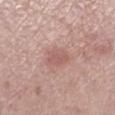– follow-up · total-body-photography surveillance lesion; no biopsy
– diameter · ≈3 mm
– acquisition · total-body-photography crop, ~15 mm field of view
– illumination · white-light
– subject · female, aged approximately 65
– location · the left lower leg
– image-analysis metrics · a lesion area of about 5.5 mm² and a shape-asymmetry score of about 0.25 (0 = symmetric); a border-irregularity index near 2.5/10, a within-lesion color-variation index near 1.5/10, and peripheral color asymmetry of about 0.5